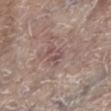Findings:
– workup · imaged on a skin check; not biopsied
– automated metrics · a lesion color around L≈50 a*≈18 b*≈20 in CIELAB and roughly 7 lightness units darker than nearby skin
– subject · female, in their mid- to late 80s or older
– location · the left lower leg
– image · ~15 mm tile from a whole-body skin photo